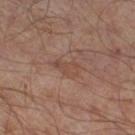biopsy status = total-body-photography surveillance lesion; no biopsy | illumination = cross-polarized illumination | lesion diameter = ≈3.5 mm | site = the right thigh | subject = male, approximately 65 years of age | image = 15 mm crop, total-body photography.